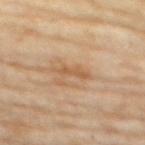notes — total-body-photography surveillance lesion; no biopsy | subject — female, in their mid-70s | anatomic site — the chest | imaging modality — ~15 mm tile from a whole-body skin photo.